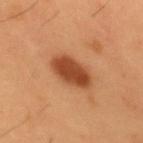biopsy_status: not biopsied; imaged during a skin examination
site: upper back
patient:
  sex: male
  age_approx: 55
lighting: cross-polarized
image:
  source: total-body photography crop
  field_of_view_mm: 15